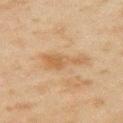Case summary:
• notes — imaged on a skin check; not biopsied
• lesion diameter — ≈4.5 mm
• body site — the left upper arm
• patient — male, aged 43–47
• TBP lesion metrics — an eccentricity of roughly 0.85 and a shape-asymmetry score of about 0.6 (0 = symmetric); a lesion color around L≈48 a*≈15 b*≈32 in CIELAB, about 7 CIELAB-L* units darker than the surrounding skin, and a normalized lesion–skin contrast near 6; a border-irregularity rating of about 6.5/10; a classifier nevus-likeness of about 0/100 and a detector confidence of about 100 out of 100 that the crop contains a lesion
• acquisition — ~15 mm crop, total-body skin-cancer survey